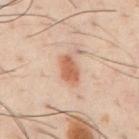| feature | finding |
|---|---|
| biopsy status | imaged on a skin check; not biopsied |
| site | the chest |
| acquisition | total-body-photography crop, ~15 mm field of view |
| lesion size | ~3.5 mm (longest diameter) |
| subject | male, about 40 years old |
| lighting | cross-polarized |